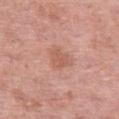Clinical impression: No biopsy was performed on this lesion — it was imaged during a full skin examination and was not determined to be concerning. Clinical summary: The lesion's longest dimension is about 2.5 mm. A female patient, aged 58–62. From the left thigh. The tile uses white-light illumination. A 15 mm close-up extracted from a 3D total-body photography capture.